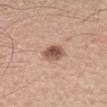workup: total-body-photography surveillance lesion; no biopsy | lighting: white-light | TBP lesion metrics: a footprint of about 5.5 mm², a shape eccentricity near 0.35, and two-axis asymmetry of about 0.2; a border-irregularity index near 1.5/10, a within-lesion color-variation index near 5/10, and radial color variation of about 2; lesion-presence confidence of about 100/100 | image source: 15 mm crop, total-body photography | patient: male, aged 58–62 | site: the arm.